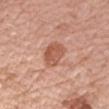Impression:
Imaged during a routine full-body skin examination; the lesion was not biopsied and no histopathology is available.
Acquisition and patient details:
Located on the right upper arm. Measured at roughly 3.5 mm in maximum diameter. A female subject, in their mid-60s. Captured under white-light illumination. A 15 mm close-up tile from a total-body photography series done for melanoma screening. An algorithmic analysis of the crop reported a lesion color around L≈56 a*≈25 b*≈31 in CIELAB, about 10 CIELAB-L* units darker than the surrounding skin, and a normalized lesion–skin contrast near 7. It also reported a nevus-likeness score of about 5/100 and lesion-presence confidence of about 100/100.